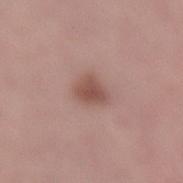Clinical impression: No biopsy was performed on this lesion — it was imaged during a full skin examination and was not determined to be concerning. Clinical summary: From the leg. A region of skin cropped from a whole-body photographic capture, roughly 15 mm wide. The subject is a female in their mid- to late 40s.